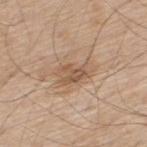Assessment:
Part of a total-body skin-imaging series; this lesion was reviewed on a skin check and was not flagged for biopsy.
Background:
The recorded lesion diameter is about 4 mm. A male subject, in their 70s. This image is a 15 mm lesion crop taken from a total-body photograph. The lesion is located on the back. Imaged with white-light lighting.